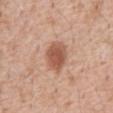This lesion was catalogued during total-body skin photography and was not selected for biopsy.
This is a white-light tile.
On the abdomen.
Cropped from a whole-body photographic skin survey; the tile spans about 15 mm.
Approximately 4 mm at its widest.
Automated image analysis of the tile measured a lesion area of about 10 mm², an outline eccentricity of about 0.65 (0 = round, 1 = elongated), and a shape-asymmetry score of about 0.15 (0 = symmetric). The analysis additionally found a border-irregularity rating of about 1.5/10, internal color variation of about 3.5 on a 0–10 scale, and peripheral color asymmetry of about 1.
A male patient, approximately 60 years of age.